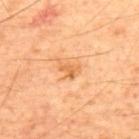Recorded during total-body skin imaging; not selected for excision or biopsy.
A roughly 15 mm field-of-view crop from a total-body skin photograph.
A male patient approximately 60 years of age.
Captured under cross-polarized illumination.
On the upper back.